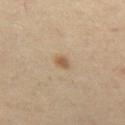{
  "lighting": "cross-polarized",
  "image": {
    "source": "total-body photography crop",
    "field_of_view_mm": 15
  },
  "site": "left thigh",
  "patient": {
    "sex": "female",
    "age_approx": 50
  },
  "lesion_size": {
    "long_diameter_mm_approx": 2.0
  }
}